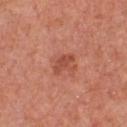follow-up = no biopsy performed (imaged during a skin exam); illumination = white-light illumination; lesion diameter = about 3 mm; acquisition = 15 mm crop, total-body photography; body site = the chest; patient = male, aged 53 to 57.